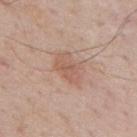{"biopsy_status": "not biopsied; imaged during a skin examination", "image": {"source": "total-body photography crop", "field_of_view_mm": 15}, "patient": {"sex": "male", "age_approx": 70}, "site": "mid back"}